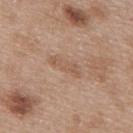This lesion was catalogued during total-body skin photography and was not selected for biopsy. Approximately 4.5 mm at its widest. A female subject aged around 40. The lesion is on the upper back. Cropped from a whole-body photographic skin survey; the tile spans about 15 mm. Captured under white-light illumination.